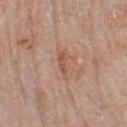The lesion was photographed on a routine skin check and not biopsied; there is no pathology result.
This is a white-light tile.
A female subject, approximately 70 years of age.
A 15 mm crop from a total-body photograph taken for skin-cancer surveillance.
Located on the head or neck.